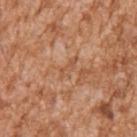The lesion was photographed on a routine skin check and not biopsied; there is no pathology result. A male patient aged approximately 45. Longest diameter approximately 3 mm. This image is a 15 mm lesion crop taken from a total-body photograph. The tile uses white-light illumination. The lesion is located on the right upper arm. An algorithmic analysis of the crop reported a footprint of about 3 mm², an eccentricity of roughly 0.95, and two-axis asymmetry of about 0.25. The analysis additionally found a nevus-likeness score of about 0/100 and a detector confidence of about 75 out of 100 that the crop contains a lesion.